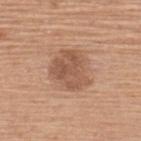Q: Was a biopsy performed?
A: catalogued during a skin exam; not biopsied
Q: What is the imaging modality?
A: ~15 mm crop, total-body skin-cancer survey
Q: Where on the body is the lesion?
A: the back
Q: How was the tile lit?
A: white-light illumination
Q: Who is the patient?
A: female, aged 58–62
Q: What did automated image analysis measure?
A: a shape eccentricity near 0.55 and a symmetry-axis asymmetry near 0.3; a mean CIELAB color near L≈54 a*≈21 b*≈31, a lesion–skin lightness drop of about 10, and a normalized lesion–skin contrast near 7; a border-irregularity index near 3/10 and a within-lesion color-variation index near 3.5/10
Q: How large is the lesion?
A: ≈4.5 mm